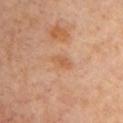biopsy status: catalogued during a skin exam; not biopsied | body site: the chest | subject: female, in their mid-60s | imaging modality: total-body-photography crop, ~15 mm field of view | illumination: cross-polarized illumination | diameter: about 2.5 mm | automated metrics: a shape eccentricity near 0.85 and a shape-asymmetry score of about 0.25 (0 = symmetric); a lesion color around L≈56 a*≈22 b*≈36 in CIELAB, roughly 7 lightness units darker than nearby skin, and a normalized border contrast of about 6; border irregularity of about 2.5 on a 0–10 scale, a within-lesion color-variation index near 1/10, and a peripheral color-asymmetry measure near 0.5; a classifier nevus-likeness of about 0/100 and a lesion-detection confidence of about 100/100.